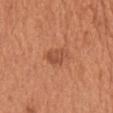The lesion was photographed on a routine skin check and not biopsied; there is no pathology result.
Imaged with white-light lighting.
Measured at roughly 2.5 mm in maximum diameter.
A region of skin cropped from a whole-body photographic capture, roughly 15 mm wide.
A male subject, approximately 65 years of age.
On the chest.